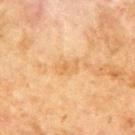notes: catalogued during a skin exam; not biopsied | automated metrics: an area of roughly 3 mm²; a lesion color around L≈55 a*≈18 b*≈38 in CIELAB, roughly 6 lightness units darker than nearby skin, and a normalized border contrast of about 5; a classifier nevus-likeness of about 0/100 and lesion-presence confidence of about 100/100 | lesion size: ≈3 mm | image: 15 mm crop, total-body photography | subject: male, aged approximately 70 | anatomic site: the upper back | tile lighting: cross-polarized.